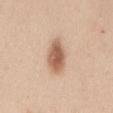Case summary:
• biopsy status · imaged on a skin check; not biopsied
• anatomic site · the mid back
• automated lesion analysis · a lesion color around L≈60 a*≈20 b*≈31 in CIELAB, a lesion–skin lightness drop of about 15, and a lesion-to-skin contrast of about 9.5 (normalized; higher = more distinct); an automated nevus-likeness rating near 100 out of 100 and a lesion-detection confidence of about 100/100
• image · ~15 mm tile from a whole-body skin photo
• patient · female, in their mid- to late 40s
• size · ~5 mm (longest diameter)
• lighting · white-light illumination